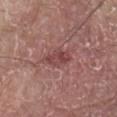Impression: Recorded during total-body skin imaging; not selected for excision or biopsy.